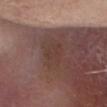tile lighting = white-light illumination; size = ≈4 mm; patient = male, about 65 years old; imaging modality = ~15 mm crop, total-body skin-cancer survey; location = the head or neck.